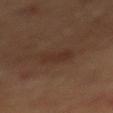Findings:
* follow-up — no biopsy performed (imaged during a skin exam)
* illumination — cross-polarized illumination
* image source — 15 mm crop, total-body photography
* site — the mid back
* patient — male, about 70 years old
* size — ~3 mm (longest diameter)
* automated metrics — a within-lesion color-variation index near 1.5/10 and radial color variation of about 0.5